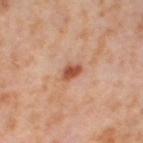  biopsy_status: not biopsied; imaged during a skin examination
  image:
    source: total-body photography crop
    field_of_view_mm: 15
  patient:
    sex: female
    age_approx: 55
  site: leg
  lesion_size:
    long_diameter_mm_approx: 2.5
  lighting: cross-polarized